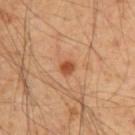Q: Was a biopsy performed?
A: imaged on a skin check; not biopsied
Q: Illumination type?
A: cross-polarized
Q: How was this image acquired?
A: total-body-photography crop, ~15 mm field of view
Q: Automated lesion metrics?
A: a border-irregularity index near 2.5/10, internal color variation of about 2 on a 0–10 scale, and a peripheral color-asymmetry measure near 1; a classifier nevus-likeness of about 80/100 and lesion-presence confidence of about 100/100
Q: Lesion location?
A: the upper back
Q: Lesion size?
A: about 2 mm
Q: Patient demographics?
A: male, aged 53 to 57